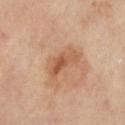Findings:
• notes — no biopsy performed (imaged during a skin exam)
• acquisition — 15 mm crop, total-body photography
• subject — female, aged 68 to 72
• location — the left lower leg